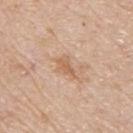Assessment: The lesion was tiled from a total-body skin photograph and was not biopsied. Clinical summary: Imaged with white-light lighting. Located on the upper back. A male subject, roughly 80 years of age. A 15 mm close-up tile from a total-body photography series done for melanoma screening. Automated tile analysis of the lesion measured a lesion area of about 4 mm² and an outline eccentricity of about 0.8 (0 = round, 1 = elongated). And it measured a classifier nevus-likeness of about 0/100 and a lesion-detection confidence of about 100/100.